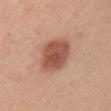<tbp_lesion>
  <biopsy_status>not biopsied; imaged during a skin examination</biopsy_status>
  <lesion_size>
    <long_diameter_mm_approx>5.0</long_diameter_mm_approx>
  </lesion_size>
  <automated_metrics>
    <cielab_L>53</cielab_L>
    <cielab_a>24</cielab_a>
    <cielab_b>29</cielab_b>
    <vs_skin_darker_L>13.0</vs_skin_darker_L>
    <vs_skin_contrast_norm>9.0</vs_skin_contrast_norm>
    <border_irregularity_0_10>1.5</border_irregularity_0_10>
    <color_variation_0_10>3.0</color_variation_0_10>
    <peripheral_color_asymmetry>1.0</peripheral_color_asymmetry>
  </automated_metrics>
  <site>chest</site>
  <patient>
    <sex>female</sex>
    <age_approx>35</age_approx>
  </patient>
  <image>
    <source>total-body photography crop</source>
    <field_of_view_mm>15</field_of_view_mm>
  </image>
  <lighting>white-light</lighting>
</tbp_lesion>